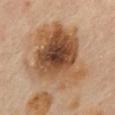Clinical impression: Imaged during a routine full-body skin examination; the lesion was not biopsied and no histopathology is available. Context: About 9 mm across. A 15 mm close-up extracted from a 3D total-body photography capture. Imaged with cross-polarized lighting. The patient is a female approximately 60 years of age. From the back. The total-body-photography lesion software estimated a lesion area of about 48 mm² and two-axis asymmetry of about 0.25. The software also gave a mean CIELAB color near L≈52 a*≈19 b*≈34, roughly 15 lightness units darker than nearby skin, and a normalized lesion–skin contrast near 10.5. It also reported border irregularity of about 3.5 on a 0–10 scale, internal color variation of about 9 on a 0–10 scale, and a peripheral color-asymmetry measure near 2.5.